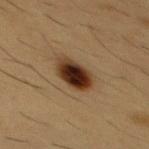Findings:
* notes: no biopsy performed (imaged during a skin exam)
* body site: the chest
* image-analysis metrics: a lesion area of about 9.5 mm², a shape eccentricity near 0.75, and a symmetry-axis asymmetry near 0.15; a border-irregularity index near 1.5/10, a within-lesion color-variation index near 8/10, and radial color variation of about 2
* lesion diameter: ≈4 mm
* patient: male, aged 53–57
* image source: ~15 mm crop, total-body skin-cancer survey